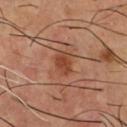Findings:
• biopsy status · catalogued during a skin exam; not biopsied
• illumination · cross-polarized illumination
• image · 15 mm crop, total-body photography
• patient · male, aged around 55
• lesion size · ~3 mm (longest diameter)
• site · the chest